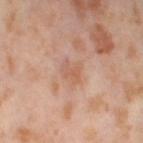Impression: No biopsy was performed on this lesion — it was imaged during a full skin examination and was not determined to be concerning. Context: An algorithmic analysis of the crop reported a footprint of about 4.5 mm², an eccentricity of roughly 0.65, and two-axis asymmetry of about 0.25. It also reported a lesion color around L≈60 a*≈22 b*≈31 in CIELAB and roughly 7 lightness units darker than nearby skin. A female subject, aged approximately 55. The lesion is located on the left thigh. Captured under cross-polarized illumination. Longest diameter approximately 2.5 mm. This image is a 15 mm lesion crop taken from a total-body photograph.